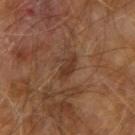Clinical impression:
This lesion was catalogued during total-body skin photography and was not selected for biopsy.